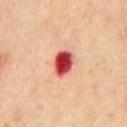The lesion was photographed on a routine skin check and not biopsied; there is no pathology result. Imaged with cross-polarized lighting. Measured at roughly 3.5 mm in maximum diameter. The lesion is on the chest. A male patient in their mid-60s. A 15 mm close-up tile from a total-body photography series done for melanoma screening.